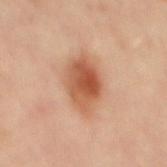The lesion was photographed on a routine skin check and not biopsied; there is no pathology result. Imaged with cross-polarized lighting. The subject is a male aged around 50. The lesion is located on the mid back. This image is a 15 mm lesion crop taken from a total-body photograph.